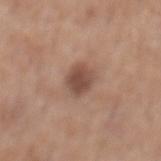Notes:
- follow-up: total-body-photography surveillance lesion; no biopsy
- patient: male, approximately 70 years of age
- body site: the mid back
- lesion diameter: about 3 mm
- automated metrics: an average lesion color of about L≈48 a*≈19 b*≈26 (CIELAB), roughly 12 lightness units darker than nearby skin, and a normalized lesion–skin contrast near 8.5; internal color variation of about 3 on a 0–10 scale and peripheral color asymmetry of about 1; a detector confidence of about 100 out of 100 that the crop contains a lesion
- imaging modality: 15 mm crop, total-body photography
- lighting: white-light